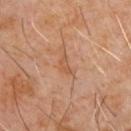<tbp_lesion>
<biopsy_status>not biopsied; imaged during a skin examination</biopsy_status>
<lighting>cross-polarized</lighting>
<site>front of the torso</site>
<patient>
  <sex>male</sex>
  <age_approx>60</age_approx>
</patient>
<image>
  <source>total-body photography crop</source>
  <field_of_view_mm>15</field_of_view_mm>
</image>
<lesion_size>
  <long_diameter_mm_approx>2.5</long_diameter_mm_approx>
</lesion_size>
</tbp_lesion>